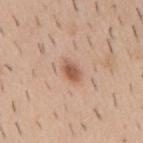Q: Was this lesion biopsied?
A: total-body-photography surveillance lesion; no biopsy
Q: Patient demographics?
A: male, approximately 40 years of age
Q: Where on the body is the lesion?
A: the mid back
Q: What kind of image is this?
A: ~15 mm tile from a whole-body skin photo